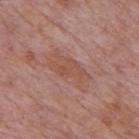Clinical impression:
Captured during whole-body skin photography for melanoma surveillance; the lesion was not biopsied.
Clinical summary:
The patient is a male aged around 75. About 6 mm across. From the mid back. This is a white-light tile. A 15 mm crop from a total-body photograph taken for skin-cancer surveillance.